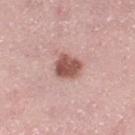From the left thigh. The lesion's longest dimension is about 3 mm. A 15 mm close-up tile from a total-body photography series done for melanoma screening. The tile uses white-light illumination. The patient is a female aged approximately 40.